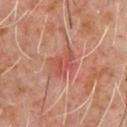Located on the chest. The tile uses cross-polarized illumination. The patient is a male about 60 years old. A close-up tile cropped from a whole-body skin photograph, about 15 mm across.